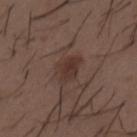Impression: The lesion was tiled from a total-body skin photograph and was not biopsied. Context: The lesion's longest dimension is about 3.5 mm. A male subject about 50 years old. On the mid back. The tile uses white-light illumination. A roughly 15 mm field-of-view crop from a total-body skin photograph. The lesion-visualizer software estimated a lesion area of about 6.5 mm² and a shape eccentricity near 0.8. The software also gave a nevus-likeness score of about 80/100 and a detector confidence of about 100 out of 100 that the crop contains a lesion.